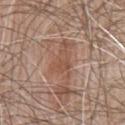location: the front of the torso
automated metrics: a footprint of about 4.5 mm², an eccentricity of roughly 0.65, and two-axis asymmetry of about 0.35; border irregularity of about 3 on a 0–10 scale and a peripheral color-asymmetry measure near 1
illumination: white-light
subject: male, aged around 80
lesion size: about 2.5 mm
acquisition: ~15 mm tile from a whole-body skin photo